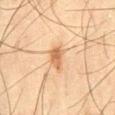Assessment: Captured during whole-body skin photography for melanoma surveillance; the lesion was not biopsied. Background: A male subject, roughly 45 years of age. A lesion tile, about 15 mm wide, cut from a 3D total-body photograph. The lesion is located on the right thigh. About 3.5 mm across. Imaged with cross-polarized lighting.